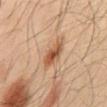The lesion is located on the mid back. Cropped from a total-body skin-imaging series; the visible field is about 15 mm. This is a cross-polarized tile. The patient is a male roughly 65 years of age.